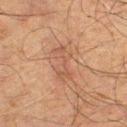Clinical impression: No biopsy was performed on this lesion — it was imaged during a full skin examination and was not determined to be concerning. Context: About 5 mm across. From the right thigh. The tile uses cross-polarized illumination. A close-up tile cropped from a whole-body skin photograph, about 15 mm across. A male patient, approximately 65 years of age.